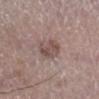Clinical impression: No biopsy was performed on this lesion — it was imaged during a full skin examination and was not determined to be concerning. Clinical summary: Measured at roughly 3.5 mm in maximum diameter. Automated tile analysis of the lesion measured an eccentricity of roughly 0.75 and a symmetry-axis asymmetry near 0.2. And it measured a border-irregularity rating of about 2/10 and internal color variation of about 4 on a 0–10 scale. And it measured a classifier nevus-likeness of about 25/100 and a lesion-detection confidence of about 100/100. A male patient, approximately 65 years of age. This is a white-light tile. Cropped from a whole-body photographic skin survey; the tile spans about 15 mm. Located on the left lower leg.